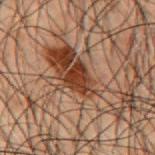<case>
<biopsy_status>not biopsied; imaged during a skin examination</biopsy_status>
<site>mid back</site>
<automated_metrics>
  <eccentricity>0.7</eccentricity>
</automated_metrics>
<image>
  <source>total-body photography crop</source>
  <field_of_view_mm>15</field_of_view_mm>
</image>
<lighting>cross-polarized</lighting>
<lesion_size>
  <long_diameter_mm_approx>3.5</long_diameter_mm_approx>
</lesion_size>
<patient>
  <sex>male</sex>
  <age_approx>50</age_approx>
</patient>
</case>